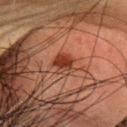This lesion was catalogued during total-body skin photography and was not selected for biopsy.
The lesion-visualizer software estimated a mean CIELAB color near L≈36 a*≈26 b*≈30, roughly 11 lightness units darker than nearby skin, and a normalized border contrast of about 9.5. The analysis additionally found a border-irregularity index near 3.5/10, internal color variation of about 3 on a 0–10 scale, and radial color variation of about 1. The software also gave a classifier nevus-likeness of about 95/100 and a detector confidence of about 100 out of 100 that the crop contains a lesion.
A roughly 15 mm field-of-view crop from a total-body skin photograph.
The subject is a male aged around 55.
Located on the head or neck.
Imaged with cross-polarized lighting.